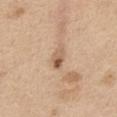Context:
This image is a 15 mm lesion crop taken from a total-body photograph. Automated tile analysis of the lesion measured an area of roughly 4.5 mm² and an outline eccentricity of about 0.85 (0 = round, 1 = elongated). The analysis additionally found a classifier nevus-likeness of about 65/100. The subject is a male about 70 years old. Longest diameter approximately 3 mm. From the abdomen.